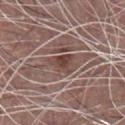The lesion was tiled from a total-body skin photograph and was not biopsied. A male patient, aged 68 to 72. About 3 mm across. The lesion is on the chest. The lesion-visualizer software estimated a lesion area of about 4.5 mm². And it measured an average lesion color of about L≈42 a*≈19 b*≈22 (CIELAB) and about 9 CIELAB-L* units darker than the surrounding skin. The analysis additionally found a border-irregularity index near 4.5/10, a within-lesion color-variation index near 2.5/10, and peripheral color asymmetry of about 0.5. The analysis additionally found lesion-presence confidence of about 75/100. The tile uses white-light illumination. A region of skin cropped from a whole-body photographic capture, roughly 15 mm wide.